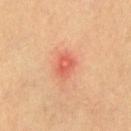Clinical impression: Recorded during total-body skin imaging; not selected for excision or biopsy. Context: A lesion tile, about 15 mm wide, cut from a 3D total-body photograph. The subject is a male approximately 60 years of age. Approximately 2.5 mm at its widest. Automated tile analysis of the lesion measured an eccentricity of roughly 0.6 and a symmetry-axis asymmetry near 0.25. The analysis additionally found a mean CIELAB color near L≈58 a*≈34 b*≈34, about 9 CIELAB-L* units darker than the surrounding skin, and a lesion-to-skin contrast of about 6 (normalized; higher = more distinct). The software also gave internal color variation of about 3 on a 0–10 scale and radial color variation of about 1. The analysis additionally found an automated nevus-likeness rating near 0 out of 100 and lesion-presence confidence of about 100/100. On the chest. This is a cross-polarized tile.